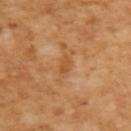Q: Was a biopsy performed?
A: catalogued during a skin exam; not biopsied
Q: Patient demographics?
A: male, in their mid-60s
Q: What kind of image is this?
A: ~15 mm crop, total-body skin-cancer survey